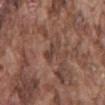Imaged during a routine full-body skin examination; the lesion was not biopsied and no histopathology is available. This image is a 15 mm lesion crop taken from a total-body photograph. The lesion is on the back. The subject is a male aged 73–77. An algorithmic analysis of the crop reported an average lesion color of about L≈41 a*≈19 b*≈23 (CIELAB) and about 7 CIELAB-L* units darker than the surrounding skin. The software also gave a within-lesion color-variation index near 3/10 and a peripheral color-asymmetry measure near 1. About 3 mm across.